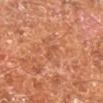Q: Was this lesion biopsied?
A: imaged on a skin check; not biopsied
Q: What are the patient's age and sex?
A: male, aged around 65
Q: Where on the body is the lesion?
A: the right lower leg
Q: What is the imaging modality?
A: ~15 mm crop, total-body skin-cancer survey